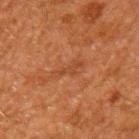| key | value |
|---|---|
| notes | no biopsy performed (imaged during a skin exam) |
| lighting | cross-polarized |
| subject | male, approximately 60 years of age |
| anatomic site | the arm |
| acquisition | 15 mm crop, total-body photography |
| lesion diameter | ~3.5 mm (longest diameter) |
| automated lesion analysis | an outline eccentricity of about 0.95 (0 = round, 1 = elongated); a lesion color around L≈35 a*≈22 b*≈30 in CIELAB, a lesion–skin lightness drop of about 5, and a lesion-to-skin contrast of about 4.5 (normalized; higher = more distinct); a classifier nevus-likeness of about 0/100 |